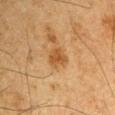<case>
  <site>left upper arm</site>
  <automated_metrics>
    <cielab_L>44</cielab_L>
    <cielab_a>18</cielab_a>
    <cielab_b>35</cielab_b>
    <vs_skin_contrast_norm>7.0</vs_skin_contrast_norm>
  </automated_metrics>
  <lighting>cross-polarized</lighting>
  <patient>
    <sex>male</sex>
    <age_approx>65</age_approx>
  </patient>
  <image>
    <source>total-body photography crop</source>
    <field_of_view_mm>15</field_of_view_mm>
  </image>
  <lesion_size>
    <long_diameter_mm_approx>3.0</long_diameter_mm_approx>
  </lesion_size>
</case>